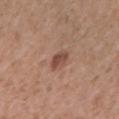follow-up = imaged on a skin check; not biopsied | acquisition = ~15 mm crop, total-body skin-cancer survey | anatomic site = the right forearm | patient = female, aged 38–42.